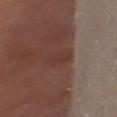notes: total-body-photography surveillance lesion; no biopsy
anatomic site: the leg
image: total-body-photography crop, ~15 mm field of view
patient: female, approximately 50 years of age
lesion diameter: ~3.5 mm (longest diameter)
lighting: cross-polarized illumination
automated metrics: a footprint of about 4.5 mm², an outline eccentricity of about 0.9 (0 = round, 1 = elongated), and a shape-asymmetry score of about 0.3 (0 = symmetric); a nevus-likeness score of about 0/100